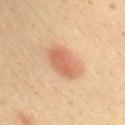The lesion was tiled from a total-body skin photograph and was not biopsied.
Located on the right upper arm.
A male patient aged 38 to 42.
This image is a 15 mm lesion crop taken from a total-body photograph.
The lesion's longest dimension is about 4.5 mm.
This is a cross-polarized tile.